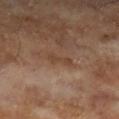notes: imaged on a skin check; not biopsied | subject: male, aged 63 to 67 | lesion diameter: ~3 mm (longest diameter) | lighting: cross-polarized illumination | image source: 15 mm crop, total-body photography.